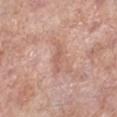Q: Was this lesion biopsied?
A: no biopsy performed (imaged during a skin exam)
Q: What is the imaging modality?
A: 15 mm crop, total-body photography
Q: Who is the patient?
A: male, aged approximately 75
Q: What is the anatomic site?
A: the left lower leg